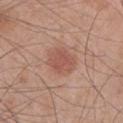• biopsy status: imaged on a skin check; not biopsied
• illumination: white-light
• site: the arm
• automated metrics: an average lesion color of about L≈53 a*≈24 b*≈27 (CIELAB), about 8 CIELAB-L* units darker than the surrounding skin, and a normalized border contrast of about 6; a border-irregularity rating of about 2/10, a within-lesion color-variation index near 2.5/10, and radial color variation of about 1; an automated nevus-likeness rating near 85 out of 100 and a detector confidence of about 100 out of 100 that the crop contains a lesion
• imaging modality: total-body-photography crop, ~15 mm field of view
• lesion diameter: about 3.5 mm
• subject: male, in their mid- to late 40s